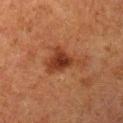Q: Was this lesion biopsied?
A: imaged on a skin check; not biopsied